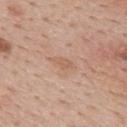workup = imaged on a skin check; not biopsied | patient = male, aged approximately 55 | anatomic site = the back | imaging modality = total-body-photography crop, ~15 mm field of view | tile lighting = white-light | diameter = about 3 mm | automated metrics = a mean CIELAB color near L≈60 a*≈20 b*≈31, about 6 CIELAB-L* units darker than the surrounding skin, and a normalized lesion–skin contrast near 5; a nevus-likeness score of about 0/100 and lesion-presence confidence of about 100/100.